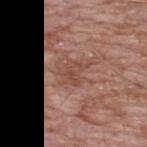workup = catalogued during a skin exam; not biopsied
lighting = white-light
subject = male, aged 58–62
lesion size = about 3 mm
body site = the upper back
acquisition = 15 mm crop, total-body photography